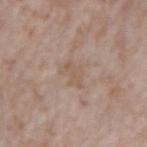This lesion was catalogued during total-body skin photography and was not selected for biopsy. A lesion tile, about 15 mm wide, cut from a 3D total-body photograph. The lesion is on the right forearm. The lesion's longest dimension is about 3.5 mm. This is a white-light tile. Automated tile analysis of the lesion measured a mean CIELAB color near L≈56 a*≈15 b*≈26, about 6 CIELAB-L* units darker than the surrounding skin, and a normalized lesion–skin contrast near 4.5. The analysis additionally found a border-irregularity index near 4/10, internal color variation of about 1.5 on a 0–10 scale, and radial color variation of about 0.5. A male patient in their mid- to late 70s.